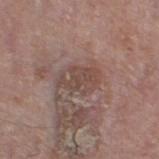follow-up: imaged on a skin check; not biopsied
anatomic site: the left thigh
acquisition: ~15 mm tile from a whole-body skin photo
tile lighting: white-light
patient: male, roughly 75 years of age
size: about 4.5 mm